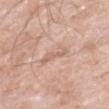Impression:
Captured during whole-body skin photography for melanoma surveillance; the lesion was not biopsied.
Acquisition and patient details:
The patient is a male roughly 75 years of age. The tile uses white-light illumination. Located on the left upper arm. A 15 mm crop from a total-body photograph taken for skin-cancer surveillance. The total-body-photography lesion software estimated a mean CIELAB color near L≈64 a*≈19 b*≈27 and a lesion–skin lightness drop of about 7. It also reported peripheral color asymmetry of about 1. And it measured a detector confidence of about 100 out of 100 that the crop contains a lesion. The lesion's longest dimension is about 3.5 mm.